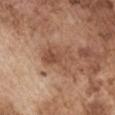Case summary:
• workup · imaged on a skin check; not biopsied
• acquisition · 15 mm crop, total-body photography
• tile lighting · white-light
• patient · male, aged 73–77
• size · ≈4.5 mm
• anatomic site · the left upper arm